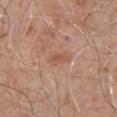Imaged during a routine full-body skin examination; the lesion was not biopsied and no histopathology is available. The lesion is on the front of the torso. The subject is a male roughly 80 years of age. Longest diameter approximately 2.5 mm. Cropped from a whole-body photographic skin survey; the tile spans about 15 mm.